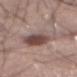The lesion was tiled from a total-body skin photograph and was not biopsied.
A male patient roughly 55 years of age.
A 15 mm close-up tile from a total-body photography series done for melanoma screening.
On the front of the torso.